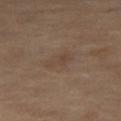Recorded during total-body skin imaging; not selected for excision or biopsy. The lesion's longest dimension is about 3 mm. A region of skin cropped from a whole-body photographic capture, roughly 15 mm wide. This is a cross-polarized tile. A female subject aged approximately 60. Located on the left leg. Automated tile analysis of the lesion measured a footprint of about 3.5 mm², an outline eccentricity of about 0.9 (0 = round, 1 = elongated), and a symmetry-axis asymmetry near 0.4. The software also gave a color-variation rating of about 1/10 and a peripheral color-asymmetry measure near 0.5.